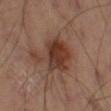The lesion was photographed on a routine skin check and not biopsied; there is no pathology result. A roughly 15 mm field-of-view crop from a total-body skin photograph. Captured under cross-polarized illumination. On the leg.